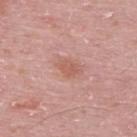Imaged during a routine full-body skin examination; the lesion was not biopsied and no histopathology is available. A male patient, approximately 50 years of age. A roughly 15 mm field-of-view crop from a total-body skin photograph. From the upper back. About 2.5 mm across.